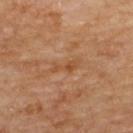Impression:
Captured during whole-body skin photography for melanoma surveillance; the lesion was not biopsied.
Clinical summary:
This image is a 15 mm lesion crop taken from a total-body photograph. Captured under cross-polarized illumination. Automated image analysis of the tile measured an automated nevus-likeness rating near 0 out of 100 and a lesion-detection confidence of about 100/100. From the upper back. The subject is a male roughly 85 years of age or older.